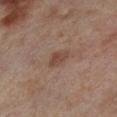The lesion was photographed on a routine skin check and not biopsied; there is no pathology result.
A female patient, about 55 years old.
The tile uses cross-polarized illumination.
The lesion is located on the right thigh.
A close-up tile cropped from a whole-body skin photograph, about 15 mm across.
Longest diameter approximately 2.5 mm.